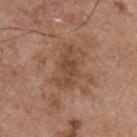notes: imaged on a skin check; not biopsied
illumination: white-light illumination
size: ~5 mm (longest diameter)
imaging modality: ~15 mm crop, total-body skin-cancer survey
automated metrics: a footprint of about 10 mm², an eccentricity of roughly 0.85, and a symmetry-axis asymmetry near 0.3; a mean CIELAB color near L≈46 a*≈20 b*≈29, roughly 8 lightness units darker than nearby skin, and a normalized lesion–skin contrast near 6.5; a nevus-likeness score of about 0/100 and lesion-presence confidence of about 100/100
patient: male, aged 53 to 57
body site: the chest